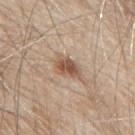This lesion was catalogued during total-body skin photography and was not selected for biopsy.
The total-body-photography lesion software estimated a lesion area of about 6 mm² and a symmetry-axis asymmetry near 0.3. The analysis additionally found a mean CIELAB color near L≈54 a*≈17 b*≈29 and a lesion–skin lightness drop of about 12. It also reported a classifier nevus-likeness of about 90/100 and lesion-presence confidence of about 100/100.
Captured under white-light illumination.
On the mid back.
The patient is a male aged 78–82.
The lesion's longest dimension is about 4 mm.
Cropped from a whole-body photographic skin survey; the tile spans about 15 mm.